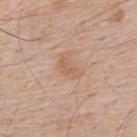The lesion-visualizer software estimated a mean CIELAB color near L≈60 a*≈20 b*≈32 and roughly 7 lightness units darker than nearby skin. The software also gave a border-irregularity rating of about 4/10, internal color variation of about 0 on a 0–10 scale, and peripheral color asymmetry of about 0. Captured under white-light illumination. A 15 mm close-up extracted from a 3D total-body photography capture. A male subject, in their 60s. The lesion is located on the upper back.